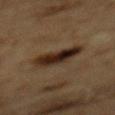notes: imaged on a skin check; not biopsied
subject: male, aged around 85 or older
lesion size: ~4.5 mm (longest diameter)
acquisition: 15 mm crop, total-body photography
lighting: cross-polarized
site: the mid back